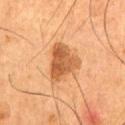The lesion was tiled from a total-body skin photograph and was not biopsied.
A close-up tile cropped from a whole-body skin photograph, about 15 mm across.
About 5.5 mm across.
Imaged with cross-polarized lighting.
Automated image analysis of the tile measured a lesion area of about 12 mm², an eccentricity of roughly 0.75, and a shape-asymmetry score of about 0.45 (0 = symmetric). It also reported a border-irregularity rating of about 4.5/10 and a peripheral color-asymmetry measure near 1.5. The analysis additionally found a nevus-likeness score of about 70/100.
A male patient aged 53 to 57.
Located on the front of the torso.